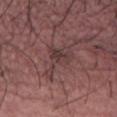Q: Was this lesion biopsied?
A: imaged on a skin check; not biopsied
Q: Patient demographics?
A: female, about 35 years old
Q: How was the tile lit?
A: white-light
Q: Where on the body is the lesion?
A: the abdomen
Q: What is the imaging modality?
A: 15 mm crop, total-body photography
Q: How large is the lesion?
A: about 4.5 mm